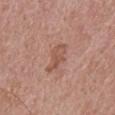follow-up = catalogued during a skin exam; not biopsied | site = the upper back | lesion size = ~4 mm (longest diameter) | image = total-body-photography crop, ~15 mm field of view | illumination = white-light | patient = male, roughly 70 years of age.